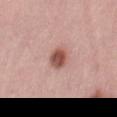Imaged during a routine full-body skin examination; the lesion was not biopsied and no histopathology is available. The recorded lesion diameter is about 3 mm. A 15 mm crop from a total-body photograph taken for skin-cancer surveillance. Captured under white-light illumination. Located on the right thigh. The lesion-visualizer software estimated a lesion area of about 6 mm² and a shape-asymmetry score of about 0.15 (0 = symmetric). It also reported a lesion color around L≈53 a*≈24 b*≈26 in CIELAB, a lesion–skin lightness drop of about 14, and a normalized border contrast of about 9.5. And it measured a border-irregularity index near 1.5/10, a color-variation rating of about 5/10, and radial color variation of about 1.5. A female patient in their mid- to late 40s.